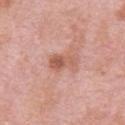The lesion was photographed on a routine skin check and not biopsied; there is no pathology result.
Imaged with white-light lighting.
From the chest.
The subject is a male aged 53 to 57.
Cropped from a total-body skin-imaging series; the visible field is about 15 mm.
Measured at roughly 3 mm in maximum diameter.
Automated tile analysis of the lesion measured a lesion area of about 5.5 mm², a shape eccentricity near 0.8, and two-axis asymmetry of about 0.35. The software also gave a mean CIELAB color near L≈57 a*≈24 b*≈29, roughly 10 lightness units darker than nearby skin, and a normalized lesion–skin contrast near 7. And it measured a border-irregularity index near 3.5/10 and peripheral color asymmetry of about 2. It also reported a nevus-likeness score of about 0/100 and a detector confidence of about 100 out of 100 that the crop contains a lesion.